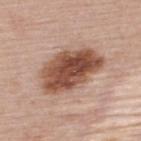<lesion>
  <biopsy_status>not biopsied; imaged during a skin examination</biopsy_status>
  <patient>
    <sex>female</sex>
    <age_approx>50</age_approx>
  </patient>
  <image>
    <source>total-body photography crop</source>
    <field_of_view_mm>15</field_of_view_mm>
  </image>
  <site>upper back</site>
  <lesion_size>
    <long_diameter_mm_approx>7.5</long_diameter_mm_approx>
  </lesion_size>
  <lighting>white-light</lighting>
</lesion>